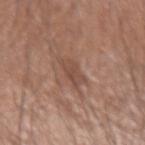  image:
    source: total-body photography crop
    field_of_view_mm: 15
  site: arm
  automated_metrics:
    area_mm2_approx: 5.0
    eccentricity: 0.8
    border_irregularity_0_10: 3.0
    color_variation_0_10: 1.5
    peripheral_color_asymmetry: 0.5
  patient:
    sex: male
    age_approx: 65